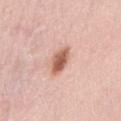Context: The subject is a female about 65 years old. The lesion is located on the mid back. A close-up tile cropped from a whole-body skin photograph, about 15 mm across.